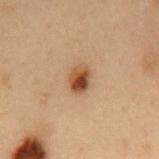Part of a total-body skin-imaging series; this lesion was reviewed on a skin check and was not flagged for biopsy. A male subject, aged around 55. A lesion tile, about 15 mm wide, cut from a 3D total-body photograph. From the mid back.